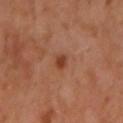<record>
<biopsy_status>not biopsied; imaged during a skin examination</biopsy_status>
<patient>
  <sex>female</sex>
  <age_approx>50</age_approx>
</patient>
<site>right forearm</site>
<image>
  <source>total-body photography crop</source>
  <field_of_view_mm>15</field_of_view_mm>
</image>
</record>